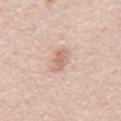<tbp_lesion>
  <biopsy_status>not biopsied; imaged during a skin examination</biopsy_status>
  <patient>
    <sex>male</sex>
    <age_approx>65</age_approx>
  </patient>
  <image>
    <source>total-body photography crop</source>
    <field_of_view_mm>15</field_of_view_mm>
  </image>
  <site>mid back</site>
</tbp_lesion>